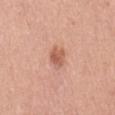{
  "biopsy_status": "not biopsied; imaged during a skin examination",
  "lesion_size": {
    "long_diameter_mm_approx": 3.0
  },
  "patient": {
    "sex": "female",
    "age_approx": 40
  },
  "image": {
    "source": "total-body photography crop",
    "field_of_view_mm": 15
  },
  "site": "mid back",
  "lighting": "white-light"
}